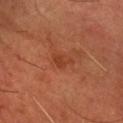Q: Was a biopsy performed?
A: imaged on a skin check; not biopsied
Q: Lesion size?
A: ≈2.5 mm
Q: What is the anatomic site?
A: the arm
Q: What lighting was used for the tile?
A: cross-polarized illumination
Q: What kind of image is this?
A: total-body-photography crop, ~15 mm field of view
Q: Who is the patient?
A: male, in their 60s